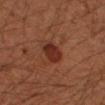Captured during whole-body skin photography for melanoma surveillance; the lesion was not biopsied. A male subject, about 50 years old. About 3 mm across. Captured under cross-polarized illumination. Located on the left forearm. A 15 mm close-up tile from a total-body photography series done for melanoma screening.